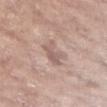This lesion was catalogued during total-body skin photography and was not selected for biopsy. An algorithmic analysis of the crop reported a shape eccentricity near 0.85 and a shape-asymmetry score of about 0.45 (0 = symmetric). It also reported a mean CIELAB color near L≈59 a*≈17 b*≈22 and about 9 CIELAB-L* units darker than the surrounding skin. And it measured a nevus-likeness score of about 0/100 and lesion-presence confidence of about 85/100. A region of skin cropped from a whole-body photographic capture, roughly 15 mm wide. Longest diameter approximately 3.5 mm. A female subject approximately 75 years of age. The lesion is located on the left forearm.